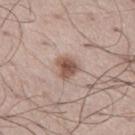| key | value |
|---|---|
| workup | catalogued during a skin exam; not biopsied |
| image-analysis metrics | a footprint of about 6 mm², an eccentricity of roughly 0.3, and two-axis asymmetry of about 0.25; an average lesion color of about L≈54 a*≈18 b*≈26 (CIELAB) and a lesion–skin lightness drop of about 12 |
| location | the left thigh |
| image | ~15 mm tile from a whole-body skin photo |
| lighting | white-light illumination |
| subject | male, about 50 years old |
| diameter | ≈3 mm |